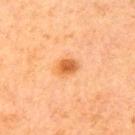patient = female, approximately 60 years of age; image source = total-body-photography crop, ~15 mm field of view; site = the right upper arm.